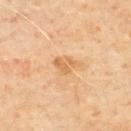Assessment:
Part of a total-body skin-imaging series; this lesion was reviewed on a skin check and was not flagged for biopsy.
Acquisition and patient details:
From the upper back. The patient is a male aged around 65. An algorithmic analysis of the crop reported a color-variation rating of about 2/10 and radial color variation of about 0.5. The software also gave a nevus-likeness score of about 0/100. A roughly 15 mm field-of-view crop from a total-body skin photograph. Imaged with cross-polarized lighting. Approximately 3 mm at its widest.